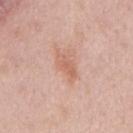The lesion was tiled from a total-body skin photograph and was not biopsied. The lesion's longest dimension is about 3.5 mm. This is a white-light tile. A male subject about 65 years old. Cropped from a total-body skin-imaging series; the visible field is about 15 mm. From the chest. Automated tile analysis of the lesion measured an area of roughly 5 mm², a shape eccentricity near 0.9, and a symmetry-axis asymmetry near 0.25.